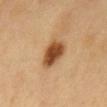{
  "biopsy_status": "not biopsied; imaged during a skin examination",
  "image": {
    "source": "total-body photography crop",
    "field_of_view_mm": 15
  },
  "lighting": "cross-polarized",
  "automated_metrics": {
    "cielab_L": 48,
    "cielab_a": 22,
    "cielab_b": 38,
    "vs_skin_darker_L": 17.0,
    "vs_skin_contrast_norm": 11.5
  },
  "lesion_size": {
    "long_diameter_mm_approx": 4.0
  },
  "patient": {
    "sex": "male",
    "age_approx": 60
  },
  "site": "abdomen"
}